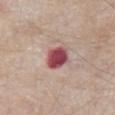  biopsy_status: not biopsied; imaged during a skin examination
  automated_metrics:
    area_mm2_approx: 7.0
    eccentricity: 0.55
    cielab_L: 48
    cielab_a: 31
    cielab_b: 19
    vs_skin_contrast_norm: 13.0
    border_irregularity_0_10: 1.5
    color_variation_0_10: 3.5
    peripheral_color_asymmetry: 1.0
    nevus_likeness_0_100: 0
    lesion_detection_confidence_0_100: 100
  image:
    source: total-body photography crop
    field_of_view_mm: 15
  site: abdomen
  patient:
    sex: male
    age_approx: 65
  lesion_size:
    long_diameter_mm_approx: 3.0